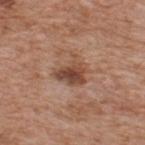{"biopsy_status": "not biopsied; imaged during a skin examination"}